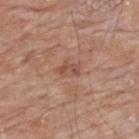A male subject roughly 60 years of age. Automated tile analysis of the lesion measured a lesion area of about 2.5 mm², an outline eccentricity of about 0.9 (0 = round, 1 = elongated), and a shape-asymmetry score of about 0.4 (0 = symmetric). The analysis additionally found a mean CIELAB color near L≈50 a*≈22 b*≈28 and a normalized lesion–skin contrast near 6. And it measured border irregularity of about 4.5 on a 0–10 scale and internal color variation of about 0 on a 0–10 scale. And it measured an automated nevus-likeness rating near 0 out of 100 and lesion-presence confidence of about 100/100. On the back. Cropped from a whole-body photographic skin survey; the tile spans about 15 mm. The tile uses white-light illumination.